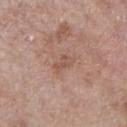workup — no biopsy performed (imaged during a skin exam) | automated lesion analysis — a mean CIELAB color near L≈53 a*≈21 b*≈27, a lesion–skin lightness drop of about 7, and a normalized lesion–skin contrast near 5; border irregularity of about 3.5 on a 0–10 scale, a within-lesion color-variation index near 0.5/10, and a peripheral color-asymmetry measure near 0 | diameter — about 2.5 mm | lighting — white-light | acquisition — total-body-photography crop, ~15 mm field of view | location — the right lower leg | subject — female, approximately 85 years of age or older.